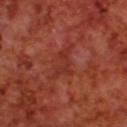Recorded during total-body skin imaging; not selected for excision or biopsy.